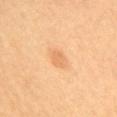workup: imaged on a skin check; not biopsied | location: the mid back | patient: female, about 65 years old | imaging modality: 15 mm crop, total-body photography.